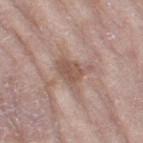Recorded during total-body skin imaging; not selected for excision or biopsy. A female subject, aged around 70. Captured under white-light illumination. This image is a 15 mm lesion crop taken from a total-body photograph. The lesion is on the right thigh. An algorithmic analysis of the crop reported a footprint of about 7.5 mm², a shape eccentricity near 0.65, and a shape-asymmetry score of about 0.45 (0 = symmetric). The analysis additionally found a mean CIELAB color near L≈54 a*≈18 b*≈25, a lesion–skin lightness drop of about 9, and a normalized lesion–skin contrast near 6.5. The analysis additionally found a border-irregularity index near 5/10 and internal color variation of about 2 on a 0–10 scale. The analysis additionally found a nevus-likeness score of about 0/100 and a detector confidence of about 95 out of 100 that the crop contains a lesion. Measured at roughly 4 mm in maximum diameter.